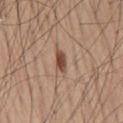lesion size: about 2.5 mm; body site: the back; acquisition: 15 mm crop, total-body photography; subject: male, aged 63–67.